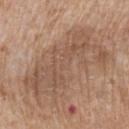Q: Was this lesion biopsied?
A: total-body-photography surveillance lesion; no biopsy
Q: How large is the lesion?
A: ≈10.5 mm
Q: What is the anatomic site?
A: the mid back
Q: Automated lesion metrics?
A: a lesion area of about 41 mm², an outline eccentricity of about 0.9 (0 = round, 1 = elongated), and a shape-asymmetry score of about 0.35 (0 = symmetric); about 8 CIELAB-L* units darker than the surrounding skin
Q: What lighting was used for the tile?
A: white-light
Q: Who is the patient?
A: male, roughly 60 years of age
Q: What kind of image is this?
A: ~15 mm crop, total-body skin-cancer survey